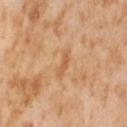| key | value |
|---|---|
| workup | no biopsy performed (imaged during a skin exam) |
| subject | female, aged approximately 55 |
| diameter | ≈3 mm |
| automated metrics | an outline eccentricity of about 0.95 (0 = round, 1 = elongated) and a symmetry-axis asymmetry near 0.35; border irregularity of about 4 on a 0–10 scale, a color-variation rating of about 0/10, and radial color variation of about 0; an automated nevus-likeness rating near 0 out of 100 and a lesion-detection confidence of about 100/100 |
| imaging modality | 15 mm crop, total-body photography |
| body site | the leg |
| illumination | cross-polarized illumination |